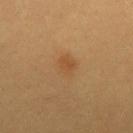Case summary:
- patient — female, aged around 30
- automated lesion analysis — a border-irregularity index near 2/10, a color-variation rating of about 2.5/10, and radial color variation of about 1
- image — total-body-photography crop, ~15 mm field of view
- body site — the back
- diameter — about 3.5 mm